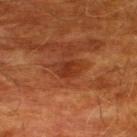Imaged during a routine full-body skin examination; the lesion was not biopsied and no histopathology is available. The tile uses cross-polarized illumination. The lesion is on the upper back. Automated tile analysis of the lesion measured a lesion area of about 5.5 mm², an eccentricity of roughly 0.75, and a symmetry-axis asymmetry near 0.3. The analysis additionally found a border-irregularity rating of about 3/10, internal color variation of about 2.5 on a 0–10 scale, and radial color variation of about 1. A male subject in their 60s. A lesion tile, about 15 mm wide, cut from a 3D total-body photograph. About 3 mm across.